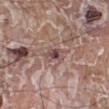<record>
  <biopsy_status>not biopsied; imaged during a skin examination</biopsy_status>
  <lighting>white-light</lighting>
  <lesion_size>
    <long_diameter_mm_approx>3.0</long_diameter_mm_approx>
  </lesion_size>
  <image>
    <source>total-body photography crop</source>
    <field_of_view_mm>15</field_of_view_mm>
  </image>
  <site>left lower leg</site>
  <patient>
    <sex>male</sex>
    <age_approx>80</age_approx>
  </patient>
</record>